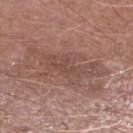No biopsy was performed on this lesion — it was imaged during a full skin examination and was not determined to be concerning.